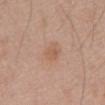  biopsy_status: not biopsied; imaged during a skin examination
  image:
    source: total-body photography crop
    field_of_view_mm: 15
  patient:
    sex: male
    age_approx: 70
  site: mid back